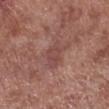| key | value |
|---|---|
| biopsy status | total-body-photography surveillance lesion; no biopsy |
| imaging modality | 15 mm crop, total-body photography |
| body site | the right lower leg |
| tile lighting | white-light |
| patient | male, in their mid-50s |
| lesion diameter | ≈3.5 mm |